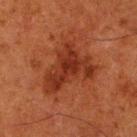Impression:
No biopsy was performed on this lesion — it was imaged during a full skin examination and was not determined to be concerning.
Image and clinical context:
A male patient, aged 78 to 82. The lesion is located on the right lower leg. Imaged with cross-polarized lighting. The total-body-photography lesion software estimated an average lesion color of about L≈26 a*≈25 b*≈28 (CIELAB) and a normalized border contrast of about 8. The software also gave a border-irregularity index near 7/10, internal color variation of about 3 on a 0–10 scale, and radial color variation of about 1. A 15 mm close-up tile from a total-body photography series done for melanoma screening. The recorded lesion diameter is about 6.5 mm.